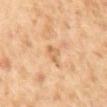Q: Was this lesion biopsied?
A: catalogued during a skin exam; not biopsied
Q: What lighting was used for the tile?
A: cross-polarized
Q: Automated lesion metrics?
A: a lesion area of about 3 mm², an eccentricity of roughly 0.85, and two-axis asymmetry of about 0.45; an average lesion color of about L≈57 a*≈18 b*≈35 (CIELAB) and a normalized border contrast of about 5.5; a color-variation rating of about 1.5/10; a classifier nevus-likeness of about 0/100 and a detector confidence of about 100 out of 100 that the crop contains a lesion
Q: What is the imaging modality?
A: ~15 mm crop, total-body skin-cancer survey
Q: What are the patient's age and sex?
A: female, aged approximately 60
Q: Where on the body is the lesion?
A: the upper back